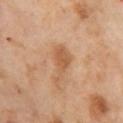Assessment: No biopsy was performed on this lesion — it was imaged during a full skin examination and was not determined to be concerning. Background: The lesion is located on the left thigh. A female patient, aged 53 to 57. Cropped from a whole-body photographic skin survey; the tile spans about 15 mm.